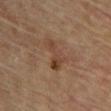biopsy status: catalogued during a skin exam; not biopsied
location: the chest
lesion size: ~4.5 mm (longest diameter)
patient: female, approximately 80 years of age
image source: ~15 mm tile from a whole-body skin photo